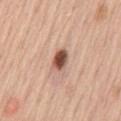| field | value |
|---|---|
| workup | no biopsy performed (imaged during a skin exam) |
| anatomic site | the back |
| patient | female, aged approximately 45 |
| image source | ~15 mm tile from a whole-body skin photo |